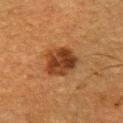| feature | finding |
|---|---|
| notes | no biopsy performed (imaged during a skin exam) |
| illumination | cross-polarized illumination |
| image-analysis metrics | a lesion color around L≈32 a*≈20 b*≈30 in CIELAB, about 11 CIELAB-L* units darker than the surrounding skin, and a lesion-to-skin contrast of about 10.5 (normalized; higher = more distinct); an automated nevus-likeness rating near 80 out of 100 and a lesion-detection confidence of about 100/100 |
| location | the left upper arm |
| imaging modality | ~15 mm tile from a whole-body skin photo |
| patient | male, roughly 50 years of age |
| lesion size | ~4 mm (longest diameter) |